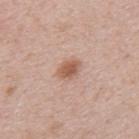Captured during whole-body skin photography for melanoma surveillance; the lesion was not biopsied. About 3 mm across. The lesion is located on the upper back. Captured under white-light illumination. A 15 mm close-up tile from a total-body photography series done for melanoma screening. The total-body-photography lesion software estimated an outline eccentricity of about 0.65 (0 = round, 1 = elongated). And it measured a lesion color around L≈58 a*≈21 b*≈29 in CIELAB, about 11 CIELAB-L* units darker than the surrounding skin, and a normalized lesion–skin contrast near 7.5. And it measured an automated nevus-likeness rating near 95 out of 100. The patient is a male aged approximately 40.